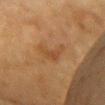Clinical impression:
Recorded during total-body skin imaging; not selected for excision or biopsy.
Acquisition and patient details:
Measured at roughly 3.5 mm in maximum diameter. The subject is a female aged 53 to 57. This is a cross-polarized tile. Automated tile analysis of the lesion measured two-axis asymmetry of about 0.65. The analysis additionally found a mean CIELAB color near L≈39 a*≈18 b*≈32, roughly 6 lightness units darker than nearby skin, and a normalized lesion–skin contrast near 5.5. A close-up tile cropped from a whole-body skin photograph, about 15 mm across. On the chest.